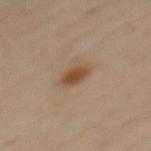biopsy status — catalogued during a skin exam; not biopsied | patient — male, in their mid-50s | acquisition — 15 mm crop, total-body photography | site — the upper back | tile lighting — cross-polarized.